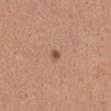• biopsy status — total-body-photography surveillance lesion; no biopsy
• subject — female, in their mid- to late 40s
• image source — 15 mm crop, total-body photography
• body site — the chest
• lighting — white-light
• automated lesion analysis — a border-irregularity rating of about 1.5/10, a color-variation rating of about 0/10, and a peripheral color-asymmetry measure near 0; an automated nevus-likeness rating near 90 out of 100 and a detector confidence of about 100 out of 100 that the crop contains a lesion